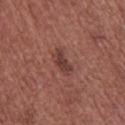<case>
  <biopsy_status>not biopsied; imaged during a skin examination</biopsy_status>
  <automated_metrics>
    <cielab_L>39</cielab_L>
    <cielab_a>22</cielab_a>
    <cielab_b>23</cielab_b>
    <vs_skin_darker_L>9.0</vs_skin_darker_L>
    <color_variation_0_10>2.5</color_variation_0_10>
    <peripheral_color_asymmetry>1.0</peripheral_color_asymmetry>
    <lesion_detection_confidence_0_100>100</lesion_detection_confidence_0_100>
  </automated_metrics>
  <site>back</site>
  <image>
    <source>total-body photography crop</source>
    <field_of_view_mm>15</field_of_view_mm>
  </image>
  <patient>
    <sex>female</sex>
    <age_approx>65</age_approx>
  </patient>
  <lesion_size>
    <long_diameter_mm_approx>3.5</long_diameter_mm_approx>
  </lesion_size>
</case>